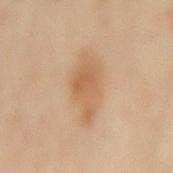This lesion was catalogued during total-body skin photography and was not selected for biopsy. The lesion is located on the mid back. A close-up tile cropped from a whole-body skin photograph, about 15 mm across. A female subject aged 58–62. Imaged with cross-polarized lighting. Approximately 6.5 mm at its widest. The lesion-visualizer software estimated a lesion color around L≈60 a*≈18 b*≈36 in CIELAB, roughly 9 lightness units darker than nearby skin, and a normalized border contrast of about 6.5. The analysis additionally found a border-irregularity rating of about 3.5/10, a within-lesion color-variation index near 3/10, and a peripheral color-asymmetry measure near 1.